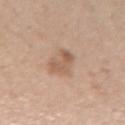Captured during whole-body skin photography for melanoma surveillance; the lesion was not biopsied. A roughly 15 mm field-of-view crop from a total-body skin photograph. Approximately 4 mm at its widest. The total-body-photography lesion software estimated a lesion color around L≈58 a*≈18 b*≈30 in CIELAB and a lesion-to-skin contrast of about 6.5 (normalized; higher = more distinct). And it measured border irregularity of about 4.5 on a 0–10 scale, a color-variation rating of about 3/10, and a peripheral color-asymmetry measure near 0.5. The analysis additionally found a classifier nevus-likeness of about 0/100 and a lesion-detection confidence of about 100/100. The lesion is on the left forearm. Imaged with white-light lighting. The subject is a female about 40 years old.